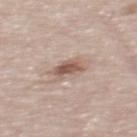Imaged during a routine full-body skin examination; the lesion was not biopsied and no histopathology is available. Cropped from a total-body skin-imaging series; the visible field is about 15 mm. The lesion's longest dimension is about 3 mm. The lesion is on the mid back. The total-body-photography lesion software estimated an average lesion color of about L≈55 a*≈18 b*≈25 (CIELAB) and about 13 CIELAB-L* units darker than the surrounding skin. The analysis additionally found a border-irregularity index near 3.5/10 and peripheral color asymmetry of about 1. The software also gave a detector confidence of about 100 out of 100 that the crop contains a lesion. A male subject, about 75 years old. Imaged with white-light lighting.